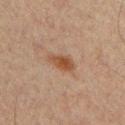Assessment:
The lesion was tiled from a total-body skin photograph and was not biopsied.
Clinical summary:
A male patient aged 58 to 62. A 15 mm close-up tile from a total-body photography series done for melanoma screening. Captured under cross-polarized illumination. Automated image analysis of the tile measured a lesion area of about 5.5 mm² and two-axis asymmetry of about 0.2. The software also gave a lesion–skin lightness drop of about 8 and a lesion-to-skin contrast of about 8 (normalized; higher = more distinct). Located on the chest. Longest diameter approximately 3.5 mm.